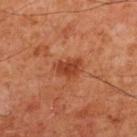notes: catalogued during a skin exam; not biopsied | tile lighting: cross-polarized illumination | anatomic site: the front of the torso | TBP lesion metrics: an area of roughly 5.5 mm², an eccentricity of roughly 0.7, and a shape-asymmetry score of about 0.35 (0 = symmetric); a lesion color around L≈38 a*≈28 b*≈33 in CIELAB and a normalized lesion–skin contrast near 8 | subject: male, approximately 60 years of age | lesion size: ~3 mm (longest diameter) | image: ~15 mm tile from a whole-body skin photo.